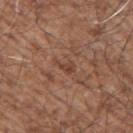Notes:
* follow-up: imaged on a skin check; not biopsied
* lesion diameter: about 2.5 mm
* automated metrics: an average lesion color of about L≈43 a*≈21 b*≈28 (CIELAB) and about 7 CIELAB-L* units darker than the surrounding skin
* patient: male, roughly 55 years of age
* image: 15 mm crop, total-body photography
* location: the right upper arm